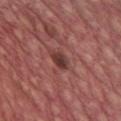Part of a total-body skin-imaging series; this lesion was reviewed on a skin check and was not flagged for biopsy. A male subject about 60 years old. This image is a 15 mm lesion crop taken from a total-body photograph. Automated tile analysis of the lesion measured an area of roughly 4 mm², an eccentricity of roughly 0.85, and a symmetry-axis asymmetry near 0.25. The software also gave about 11 CIELAB-L* units darker than the surrounding skin and a normalized border contrast of about 9. And it measured radial color variation of about 1. This is a white-light tile. From the front of the torso. The lesion's longest dimension is about 3 mm.